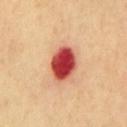Impression: No biopsy was performed on this lesion — it was imaged during a full skin examination and was not determined to be concerning. Context: From the chest. A region of skin cropped from a whole-body photographic capture, roughly 15 mm wide. The subject is a male roughly 70 years of age. This is a cross-polarized tile.